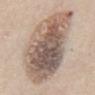Q: Was a biopsy performed?
A: catalogued during a skin exam; not biopsied
Q: Patient demographics?
A: male, roughly 60 years of age
Q: Lesion size?
A: ~10 mm (longest diameter)
Q: What lighting was used for the tile?
A: white-light illumination
Q: What is the anatomic site?
A: the abdomen
Q: What kind of image is this?
A: total-body-photography crop, ~15 mm field of view